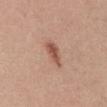workup: catalogued during a skin exam; not biopsied
image source: ~15 mm tile from a whole-body skin photo
site: the back
patient: female, roughly 35 years of age